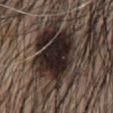Clinical impression:
The lesion was tiled from a total-body skin photograph and was not biopsied.
Background:
A male subject in their mid- to late 50s. A 15 mm close-up tile from a total-body photography series done for melanoma screening. Imaged with white-light lighting. Automated image analysis of the tile measured a mean CIELAB color near L≈20 a*≈10 b*≈13, roughly 18 lightness units darker than nearby skin, and a normalized lesion–skin contrast near 19. The analysis additionally found a peripheral color-asymmetry measure near 1.5. The lesion is located on the abdomen.